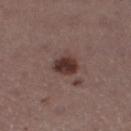The lesion was tiled from a total-body skin photograph and was not biopsied.
On the right thigh.
The recorded lesion diameter is about 2.5 mm.
A female subject roughly 55 years of age.
Captured under white-light illumination.
The total-body-photography lesion software estimated an area of roughly 5.5 mm², a shape eccentricity near 0.55, and a symmetry-axis asymmetry near 0.2. And it measured a mean CIELAB color near L≈33 a*≈19 b*≈20. The software also gave a nevus-likeness score of about 95/100 and lesion-presence confidence of about 100/100.
A region of skin cropped from a whole-body photographic capture, roughly 15 mm wide.